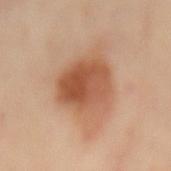The lesion was photographed on a routine skin check and not biopsied; there is no pathology result.
The lesion is located on the mid back.
Automated tile analysis of the lesion measured a footprint of about 22 mm², a shape eccentricity near 0.5, and a shape-asymmetry score of about 0.2 (0 = symmetric). It also reported a border-irregularity index near 2/10. The analysis additionally found a classifier nevus-likeness of about 95/100 and a detector confidence of about 100 out of 100 that the crop contains a lesion.
A female subject roughly 60 years of age.
Cropped from a total-body skin-imaging series; the visible field is about 15 mm.